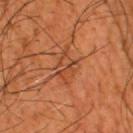Recorded during total-body skin imaging; not selected for excision or biopsy.
A male patient roughly 45 years of age.
Captured under cross-polarized illumination.
Cropped from a total-body skin-imaging series; the visible field is about 15 mm.
From the head or neck.